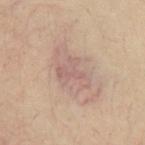| key | value |
|---|---|
| notes | no biopsy performed (imaged during a skin exam) |
| patient | male, aged around 30 |
| image | ~15 mm crop, total-body skin-cancer survey |
| location | the front of the torso |